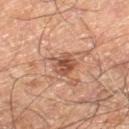Q: Was a biopsy performed?
A: imaged on a skin check; not biopsied
Q: What is the lesion's diameter?
A: about 3.5 mm
Q: Where on the body is the lesion?
A: the left thigh
Q: What is the imaging modality?
A: ~15 mm crop, total-body skin-cancer survey
Q: How was the tile lit?
A: cross-polarized
Q: Patient demographics?
A: male, aged 58–62